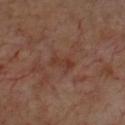Assessment:
The lesion was photographed on a routine skin check and not biopsied; there is no pathology result.
Image and clinical context:
Automated tile analysis of the lesion measured a shape eccentricity near 0.9 and two-axis asymmetry of about 0.3. And it measured a lesion–skin lightness drop of about 5. A 15 mm close-up extracted from a 3D total-body photography capture. Captured under cross-polarized illumination. A male patient aged approximately 70. On the chest. Approximately 3 mm at its widest.